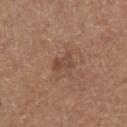notes: total-body-photography surveillance lesion; no biopsy | acquisition: 15 mm crop, total-body photography | subject: male, aged approximately 75 | lesion size: ≈2.5 mm | anatomic site: the right lower leg | tile lighting: white-light.